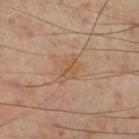{"biopsy_status": "not biopsied; imaged during a skin examination", "site": "right lower leg", "image": {"source": "total-body photography crop", "field_of_view_mm": 15}, "patient": {"sex": "male", "age_approx": 55}, "lighting": "cross-polarized"}